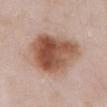{
  "biopsy_status": "not biopsied; imaged during a skin examination",
  "automated_metrics": {
    "border_irregularity_0_10": 2.5,
    "color_variation_0_10": 8.5,
    "peripheral_color_asymmetry": 3.0
  },
  "site": "abdomen",
  "patient": {
    "sex": "male",
    "age_approx": 55
  },
  "lesion_size": {
    "long_diameter_mm_approx": 7.5
  },
  "image": {
    "source": "total-body photography crop",
    "field_of_view_mm": 15
  }
}